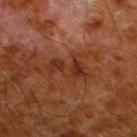This lesion was catalogued during total-body skin photography and was not selected for biopsy. A male subject aged 78 to 82. Cropped from a total-body skin-imaging series; the visible field is about 15 mm. Located on the left lower leg.